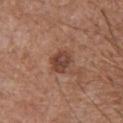The lesion was photographed on a routine skin check and not biopsied; there is no pathology result.
The subject is a male approximately 65 years of age.
The lesion is on the chest.
A close-up tile cropped from a whole-body skin photograph, about 15 mm across.
The lesion-visualizer software estimated an area of roughly 6 mm², a shape eccentricity near 0.55, and two-axis asymmetry of about 0.25. And it measured a classifier nevus-likeness of about 5/100.